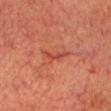Captured during whole-body skin photography for melanoma surveillance; the lesion was not biopsied. A close-up tile cropped from a whole-body skin photograph, about 15 mm across. A male subject, aged approximately 75. The lesion is located on the head or neck.